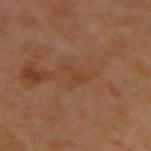Captured under cross-polarized illumination.
The lesion's longest dimension is about 3 mm.
Automated tile analysis of the lesion measured a border-irregularity rating of about 5/10, a within-lesion color-variation index near 0.5/10, and a peripheral color-asymmetry measure near 0. The analysis additionally found an automated nevus-likeness rating near 0 out of 100 and a detector confidence of about 100 out of 100 that the crop contains a lesion.
A male patient aged approximately 65.
From the left arm.
A 15 mm crop from a total-body photograph taken for skin-cancer surveillance.